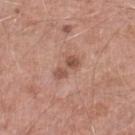No biopsy was performed on this lesion — it was imaged during a full skin examination and was not determined to be concerning.
Automated tile analysis of the lesion measured an area of roughly 4 mm², an outline eccentricity of about 0.9 (0 = round, 1 = elongated), and a shape-asymmetry score of about 0.25 (0 = symmetric). The analysis additionally found a lesion color around L≈52 a*≈22 b*≈28 in CIELAB and a normalized border contrast of about 7.5.
The lesion is located on the left lower leg.
A region of skin cropped from a whole-body photographic capture, roughly 15 mm wide.
About 3.5 mm across.
The subject is a male aged approximately 50.